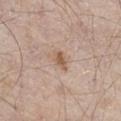The lesion was tiled from a total-body skin photograph and was not biopsied. The subject is a male roughly 65 years of age. A 15 mm close-up tile from a total-body photography series done for melanoma screening. The lesion-visualizer software estimated an area of roughly 3 mm² and a symmetry-axis asymmetry near 0.3. It also reported an average lesion color of about L≈57 a*≈18 b*≈29 (CIELAB), a lesion–skin lightness drop of about 10, and a lesion-to-skin contrast of about 7.5 (normalized; higher = more distinct). It also reported a nevus-likeness score of about 70/100 and lesion-presence confidence of about 100/100. The lesion is located on the leg. The recorded lesion diameter is about 2.5 mm.